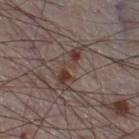Part of a total-body skin-imaging series; this lesion was reviewed on a skin check and was not flagged for biopsy.
This is a white-light tile.
Cropped from a whole-body photographic skin survey; the tile spans about 15 mm.
The patient is a male aged 73 to 77.
The recorded lesion diameter is about 5.5 mm.
Located on the right thigh.